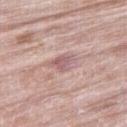Captured during whole-body skin photography for melanoma surveillance; the lesion was not biopsied.